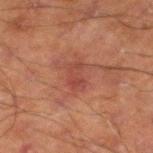No biopsy was performed on this lesion — it was imaged during a full skin examination and was not determined to be concerning.
A roughly 15 mm field-of-view crop from a total-body skin photograph.
From the leg.
The patient is a male about 70 years old.